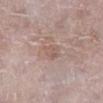* follow-up: no biopsy performed (imaged during a skin exam)
* TBP lesion metrics: a shape eccentricity near 0.7 and two-axis asymmetry of about 0.2; roughly 6 lightness units darker than nearby skin and a lesion-to-skin contrast of about 5 (normalized; higher = more distinct)
* location: the right lower leg
* image source: ~15 mm crop, total-body skin-cancer survey
* patient: female, aged approximately 70